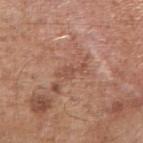Captured during whole-body skin photography for melanoma surveillance; the lesion was not biopsied.
The lesion's longest dimension is about 3.5 mm.
On the right upper arm.
The subject is a male approximately 65 years of age.
The total-body-photography lesion software estimated an area of roughly 3 mm², an outline eccentricity of about 0.95 (0 = round, 1 = elongated), and two-axis asymmetry of about 0.6. It also reported a border-irregularity rating of about 7.5/10 and peripheral color asymmetry of about 0. It also reported a nevus-likeness score of about 0/100.
A 15 mm crop from a total-body photograph taken for skin-cancer surveillance.